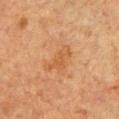| feature | finding |
|---|---|
| notes | total-body-photography surveillance lesion; no biopsy |
| image | 15 mm crop, total-body photography |
| patient | male, roughly 50 years of age |
| TBP lesion metrics | a footprint of about 5 mm², an eccentricity of roughly 0.9, and two-axis asymmetry of about 0.55; a mean CIELAB color near L≈44 a*≈20 b*≈34 and roughly 6 lightness units darker than nearby skin; a border-irregularity rating of about 6.5/10 and a peripheral color-asymmetry measure near 0.5 |
| size | ≈4 mm |
| lighting | cross-polarized |
| site | the front of the torso |